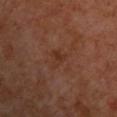Clinical impression:
Recorded during total-body skin imaging; not selected for excision or biopsy.
Image and clinical context:
Automated tile analysis of the lesion measured a lesion area of about 3 mm². The software also gave a mean CIELAB color near L≈31 a*≈20 b*≈27, about 5 CIELAB-L* units darker than the surrounding skin, and a normalized border contrast of about 6. And it measured internal color variation of about 0.5 on a 0–10 scale and radial color variation of about 0. Imaged with cross-polarized lighting. A 15 mm crop from a total-body photograph taken for skin-cancer surveillance. The lesion is located on the upper back. The patient is a male about 70 years old. Approximately 2.5 mm at its widest.